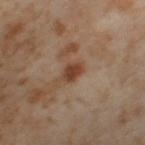notes=total-body-photography surveillance lesion; no biopsy
location=the left thigh
illumination=cross-polarized
patient=female, in their mid-50s
size=≈2.5 mm
imaging modality=15 mm crop, total-body photography